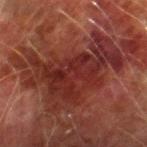Captured during whole-body skin photography for melanoma surveillance; the lesion was not biopsied. A 15 mm close-up extracted from a 3D total-body photography capture. Approximately 10.5 mm at its widest. The subject is a male aged 73–77. This is a cross-polarized tile. Automated tile analysis of the lesion measured an area of roughly 43 mm² and a shape-asymmetry score of about 0.5 (0 = symmetric). And it measured a border-irregularity rating of about 8.5/10 and a color-variation rating of about 4.5/10. The lesion is on the right thigh.